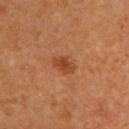Q: Was this lesion biopsied?
A: catalogued during a skin exam; not biopsied
Q: What did automated image analysis measure?
A: a color-variation rating of about 2.5/10 and a peripheral color-asymmetry measure near 0.5; a classifier nevus-likeness of about 90/100
Q: How was this image acquired?
A: ~15 mm crop, total-body skin-cancer survey
Q: Lesion size?
A: ~3 mm (longest diameter)
Q: Lesion location?
A: the chest
Q: Who is the patient?
A: male, aged approximately 60
Q: What lighting was used for the tile?
A: cross-polarized illumination